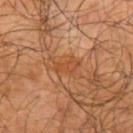Clinical impression:
This lesion was catalogued during total-body skin photography and was not selected for biopsy.
Background:
Cropped from a whole-body photographic skin survey; the tile spans about 15 mm. Located on the left upper arm. An algorithmic analysis of the crop reported about 6 CIELAB-L* units darker than the surrounding skin. Approximately 3 mm at its widest. Captured under cross-polarized illumination. The patient is a male in their mid- to late 60s.